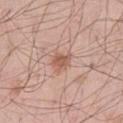The lesion was photographed on a routine skin check and not biopsied; there is no pathology result.
Imaged with white-light lighting.
A male subject about 55 years old.
A lesion tile, about 15 mm wide, cut from a 3D total-body photograph.
The recorded lesion diameter is about 3 mm.
The lesion is on the left thigh.